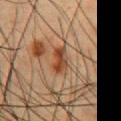Imaged during a routine full-body skin examination; the lesion was not biopsied and no histopathology is available.
The tile uses cross-polarized illumination.
Measured at roughly 3.5 mm in maximum diameter.
Located on the chest.
A region of skin cropped from a whole-body photographic capture, roughly 15 mm wide.
The subject is a male aged 48 to 52.
Automated tile analysis of the lesion measured a shape-asymmetry score of about 0.25 (0 = symmetric). The software also gave an average lesion color of about L≈36 a*≈19 b*≈28 (CIELAB), roughly 9 lightness units darker than nearby skin, and a normalized lesion–skin contrast near 8.5. The analysis additionally found a nevus-likeness score of about 45/100 and a lesion-detection confidence of about 90/100.